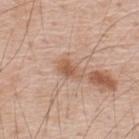Assessment: The lesion was tiled from a total-body skin photograph and was not biopsied. Context: Automated tile analysis of the lesion measured a lesion area of about 5.5 mm², an outline eccentricity of about 0.8 (0 = round, 1 = elongated), and a symmetry-axis asymmetry near 0.3. It also reported a lesion–skin lightness drop of about 9 and a normalized lesion–skin contrast near 6.5. The analysis additionally found a border-irregularity index near 3/10, internal color variation of about 3 on a 0–10 scale, and peripheral color asymmetry of about 1. The subject is a male aged approximately 50. A 15 mm close-up extracted from a 3D total-body photography capture. This is a white-light tile. On the upper back. The lesion's longest dimension is about 3.5 mm.